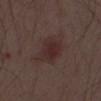Part of a total-body skin-imaging series; this lesion was reviewed on a skin check and was not flagged for biopsy.
The subject is a male aged around 55.
From the chest.
A lesion tile, about 15 mm wide, cut from a 3D total-body photograph.
The lesion's longest dimension is about 4 mm.
Imaged with white-light lighting.